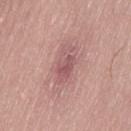Notes:
- follow-up — catalogued during a skin exam; not biopsied
- image — total-body-photography crop, ~15 mm field of view
- lesion diameter — ~4.5 mm (longest diameter)
- lighting — white-light
- anatomic site — the leg
- patient — male, aged around 50